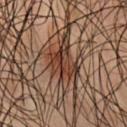Case summary:
– biopsy status — catalogued during a skin exam; not biopsied
– tile lighting — cross-polarized
– diameter — ≈4 mm
– location — the chest
– subject — male, roughly 45 years of age
– imaging modality — 15 mm crop, total-body photography